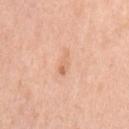Assessment:
Captured during whole-body skin photography for melanoma surveillance; the lesion was not biopsied.
Context:
An algorithmic analysis of the crop reported a lesion–skin lightness drop of about 8 and a normalized lesion–skin contrast near 5.5. And it measured border irregularity of about 4 on a 0–10 scale and a color-variation rating of about 3/10. Cropped from a whole-body photographic skin survey; the tile spans about 15 mm. The lesion is on the left upper arm. Imaged with white-light lighting. Approximately 3 mm at its widest. A female subject, aged approximately 60.